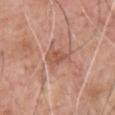This lesion was catalogued during total-body skin photography and was not selected for biopsy.
A male subject, aged 58–62.
Longest diameter approximately 3 mm.
A 15 mm close-up extracted from a 3D total-body photography capture.
From the front of the torso.